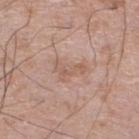<case>
  <biopsy_status>not biopsied; imaged during a skin examination</biopsy_status>
  <patient>
    <sex>male</sex>
    <age_approx>60</age_approx>
  </patient>
  <image>
    <source>total-body photography crop</source>
    <field_of_view_mm>15</field_of_view_mm>
  </image>
  <automated_metrics>
    <eccentricity>0.85</eccentricity>
    <cielab_L>58</cielab_L>
    <cielab_a>19</cielab_a>
    <cielab_b>27</cielab_b>
    <vs_skin_darker_L>7.0</vs_skin_darker_L>
    <vs_skin_contrast_norm>5.0</vs_skin_contrast_norm>
    <border_irregularity_0_10>6.5</border_irregularity_0_10>
    <color_variation_0_10>3.0</color_variation_0_10>
    <peripheral_color_asymmetry>1.0</peripheral_color_asymmetry>
  </automated_metrics>
  <lighting>white-light</lighting>
  <site>right lower leg</site>
</case>